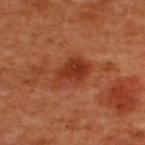Case summary:
* follow-up — imaged on a skin check; not biopsied
* automated metrics — an average lesion color of about L≈38 a*≈31 b*≈35 (CIELAB), roughly 10 lightness units darker than nearby skin, and a normalized border contrast of about 8; a border-irregularity rating of about 2.5/10, internal color variation of about 4.5 on a 0–10 scale, and a peripheral color-asymmetry measure near 1.5
* location — the upper back
* subject — male, roughly 50 years of age
* diameter — about 4.5 mm
* image source — total-body-photography crop, ~15 mm field of view
* tile lighting — cross-polarized illumination